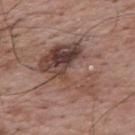Impression: This lesion was catalogued during total-body skin photography and was not selected for biopsy. Context: Approximately 8 mm at its widest. This is a white-light tile. This image is a 15 mm lesion crop taken from a total-body photograph. A male subject about 70 years old. The lesion is located on the upper back.